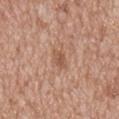The lesion was tiled from a total-body skin photograph and was not biopsied. Captured under white-light illumination. The patient is a male in their mid- to late 60s. A 15 mm close-up extracted from a 3D total-body photography capture. The recorded lesion diameter is about 3 mm. Located on the mid back. An algorithmic analysis of the crop reported a border-irregularity index near 2.5/10, a color-variation rating of about 1.5/10, and a peripheral color-asymmetry measure near 0.5. The analysis additionally found an automated nevus-likeness rating near 0 out of 100 and a lesion-detection confidence of about 100/100.